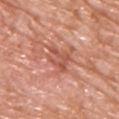<tbp_lesion>
  <patient>
    <sex>male</sex>
    <age_approx>75</age_approx>
  </patient>
  <site>upper back</site>
  <image>
    <source>total-body photography crop</source>
    <field_of_view_mm>15</field_of_view_mm>
  </image>
  <lesion_size>
    <long_diameter_mm_approx>4.0</long_diameter_mm_approx>
  </lesion_size>
</tbp_lesion>